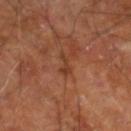{"biopsy_status": "not biopsied; imaged during a skin examination", "lighting": "cross-polarized", "patient": {"sex": "male", "age_approx": 60}, "site": "leg", "image": {"source": "total-body photography crop", "field_of_view_mm": 15}}